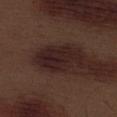biopsy status: catalogued during a skin exam; not biopsied
size: ≈6 mm
tile lighting: white-light illumination
patient: male, aged approximately 70
automated lesion analysis: a shape eccentricity near 0.85 and a symmetry-axis asymmetry near 0.35; an average lesion color of about L≈21 a*≈17 b*≈16 (CIELAB), a lesion–skin lightness drop of about 8, and a lesion-to-skin contrast of about 11 (normalized; higher = more distinct); a lesion-detection confidence of about 100/100
acquisition: total-body-photography crop, ~15 mm field of view
anatomic site: the left thigh